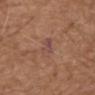{"biopsy_status": "not biopsied; imaged during a skin examination", "patient": {"sex": "male", "age_approx": 75}, "lighting": "white-light", "automated_metrics": {"border_irregularity_0_10": 3.0, "color_variation_0_10": 4.5, "peripheral_color_asymmetry": 1.5, "nevus_likeness_0_100": 0, "lesion_detection_confidence_0_100": 100}, "lesion_size": {"long_diameter_mm_approx": 3.0}, "image": {"source": "total-body photography crop", "field_of_view_mm": 15}, "site": "upper back"}